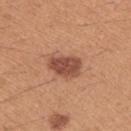workup = total-body-photography surveillance lesion; no biopsy
location = the left upper arm
image-analysis metrics = a footprint of about 10 mm² and a symmetry-axis asymmetry near 0.2; a border-irregularity rating of about 2/10, internal color variation of about 3 on a 0–10 scale, and a peripheral color-asymmetry measure near 1
lesion size = about 4.5 mm
patient = female, about 40 years old
lighting = white-light
imaging modality = ~15 mm crop, total-body skin-cancer survey